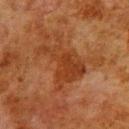Clinical impression: No biopsy was performed on this lesion — it was imaged during a full skin examination and was not determined to be concerning. Clinical summary: About 6 mm across. Imaged with cross-polarized lighting. A male patient, aged 78 to 82. From the upper back. A close-up tile cropped from a whole-body skin photograph, about 15 mm across. Automated image analysis of the tile measured a footprint of about 16 mm², an outline eccentricity of about 0.8 (0 = round, 1 = elongated), and a shape-asymmetry score of about 0.55 (0 = symmetric). It also reported border irregularity of about 7.5 on a 0–10 scale and a within-lesion color-variation index near 3/10.